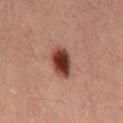notes: total-body-photography surveillance lesion; no biopsy
tile lighting: cross-polarized
acquisition: 15 mm crop, total-body photography
site: the back
subject: male, about 30 years old